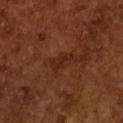Q: Was this lesion biopsied?
A: imaged on a skin check; not biopsied
Q: Lesion size?
A: ~3.5 mm (longest diameter)
Q: How was this image acquired?
A: ~15 mm tile from a whole-body skin photo
Q: Illumination type?
A: cross-polarized
Q: What are the patient's age and sex?
A: male, about 65 years old